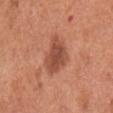Clinical summary:
An algorithmic analysis of the crop reported an average lesion color of about L≈50 a*≈26 b*≈32 (CIELAB) and roughly 11 lightness units darker than nearby skin. It also reported a border-irregularity rating of about 3/10, internal color variation of about 3 on a 0–10 scale, and peripheral color asymmetry of about 1. A roughly 15 mm field-of-view crop from a total-body skin photograph. About 4.5 mm across. The lesion is located on the chest. Captured under white-light illumination. A male subject about 65 years old.